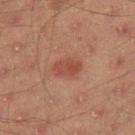Assessment:
The lesion was photographed on a routine skin check and not biopsied; there is no pathology result.
Context:
The lesion's longest dimension is about 3 mm. A 15 mm close-up tile from a total-body photography series done for melanoma screening. From the right thigh. A male patient, roughly 45 years of age. This is a cross-polarized tile.